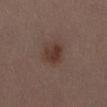The lesion was photographed on a routine skin check and not biopsied; there is no pathology result.
The subject is a female roughly 30 years of age.
Cropped from a whole-body photographic skin survey; the tile spans about 15 mm.
The lesion is on the abdomen.
Captured under white-light illumination.
The total-body-photography lesion software estimated an eccentricity of roughly 0.4 and a shape-asymmetry score of about 0.2 (0 = symmetric). The software also gave about 8 CIELAB-L* units darker than the surrounding skin and a normalized border contrast of about 7.5.
Measured at roughly 3 mm in maximum diameter.